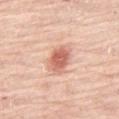<lesion>
  <biopsy_status>not biopsied; imaged during a skin examination</biopsy_status>
  <site>right thigh</site>
  <automated_metrics>
    <area_mm2_approx>7.0</area_mm2_approx>
    <eccentricity>0.65</eccentricity>
    <cielab_L>63</cielab_L>
    <cielab_a>27</cielab_a>
    <cielab_b>30</cielab_b>
    <vs_skin_contrast_norm>8.5</vs_skin_contrast_norm>
  </automated_metrics>
  <patient>
    <sex>male</sex>
    <age_approx>80</age_approx>
  </patient>
  <image>
    <source>total-body photography crop</source>
    <field_of_view_mm>15</field_of_view_mm>
  </image>
  <lesion_size>
    <long_diameter_mm_approx>3.5</long_diameter_mm_approx>
  </lesion_size>
</lesion>